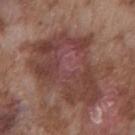notes — no biopsy performed (imaged during a skin exam) | tile lighting — white-light | anatomic site — the front of the torso | patient — male, aged 73–77 | lesion diameter — about 10 mm | image source — total-body-photography crop, ~15 mm field of view.